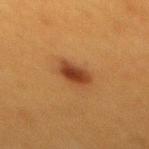{
  "biopsy_status": "not biopsied; imaged during a skin examination",
  "lighting": "cross-polarized",
  "image": {
    "source": "total-body photography crop",
    "field_of_view_mm": 15
  },
  "patient": {
    "sex": "female",
    "age_approx": 40
  },
  "automated_metrics": {
    "eccentricity": 0.8,
    "shape_asymmetry": 0.2,
    "cielab_L": 34,
    "cielab_a": 22,
    "cielab_b": 31,
    "vs_skin_darker_L": 11.0,
    "vs_skin_contrast_norm": 10.0,
    "border_irregularity_0_10": 2.0,
    "color_variation_0_10": 3.5,
    "peripheral_color_asymmetry": 1.0,
    "nevus_likeness_0_100": 100,
    "lesion_detection_confidence_0_100": 100
  },
  "lesion_size": {
    "long_diameter_mm_approx": 3.5
  },
  "site": "mid back"
}